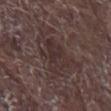The lesion was photographed on a routine skin check and not biopsied; there is no pathology result.
Measured at roughly 7.5 mm in maximum diameter.
A close-up tile cropped from a whole-body skin photograph, about 15 mm across.
The total-body-photography lesion software estimated an automated nevus-likeness rating near 0 out of 100 and a lesion-detection confidence of about 50/100.
A male subject about 65 years old.
On the arm.